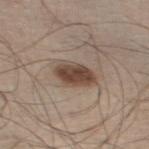{
  "biopsy_status": "not biopsied; imaged during a skin examination",
  "patient": {
    "sex": "male",
    "age_approx": 60
  },
  "lesion_size": {
    "long_diameter_mm_approx": 4.0
  },
  "site": "left thigh",
  "automated_metrics": {
    "nevus_likeness_0_100": 100,
    "lesion_detection_confidence_0_100": 100
  },
  "image": {
    "source": "total-body photography crop",
    "field_of_view_mm": 15
  },
  "lighting": "white-light"
}